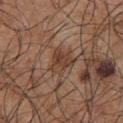Imaged with white-light lighting.
A 15 mm crop from a total-body photograph taken for skin-cancer surveillance.
From the chest.
Automated tile analysis of the lesion measured a footprint of about 6.5 mm², an outline eccentricity of about 0.6 (0 = round, 1 = elongated), and a shape-asymmetry score of about 0.45 (0 = symmetric). It also reported a lesion color around L≈41 a*≈19 b*≈27 in CIELAB, a lesion–skin lightness drop of about 9, and a normalized lesion–skin contrast near 7. The software also gave a classifier nevus-likeness of about 5/100.
The subject is a male aged approximately 55.
The recorded lesion diameter is about 3.5 mm.